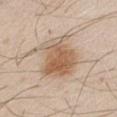site=the right upper arm
imaging modality=~15 mm crop, total-body skin-cancer survey
patient=male, approximately 30 years of age